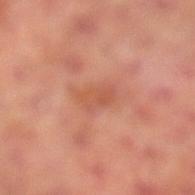notes=catalogued during a skin exam; not biopsied
anatomic site=the left lower leg
TBP lesion metrics=an average lesion color of about L≈53 a*≈27 b*≈33 (CIELAB), roughly 7 lightness units darker than nearby skin, and a normalized border contrast of about 5
subject=male, aged around 70
image=~15 mm crop, total-body skin-cancer survey